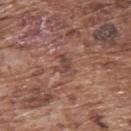biopsy_status: not biopsied; imaged during a skin examination
site: back
image:
  source: total-body photography crop
  field_of_view_mm: 15
automated_metrics:
  area_mm2_approx: 4.5
  eccentricity: 0.7
  shape_asymmetry: 0.45
  border_irregularity_0_10: 5.5
  color_variation_0_10: 3.0
  peripheral_color_asymmetry: 1.0
patient:
  sex: male
  age_approx: 75
lesion_size:
  long_diameter_mm_approx: 3.0
lighting: white-light